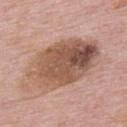Q: Is there a histopathology result?
A: catalogued during a skin exam; not biopsied
Q: What kind of image is this?
A: ~15 mm crop, total-body skin-cancer survey
Q: Who is the patient?
A: male, aged 73 to 77
Q: How large is the lesion?
A: ≈9 mm
Q: What is the anatomic site?
A: the upper back
Q: Illumination type?
A: white-light